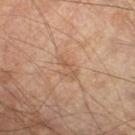The lesion was photographed on a routine skin check and not biopsied; there is no pathology result.
The lesion is on the right lower leg.
A male patient, aged 63 to 67.
This is a cross-polarized tile.
The recorded lesion diameter is about 3 mm.
This image is a 15 mm lesion crop taken from a total-body photograph.